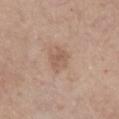No biopsy was performed on this lesion — it was imaged during a full skin examination and was not determined to be concerning.
On the chest.
The subject is a male aged approximately 60.
Approximately 3 mm at its widest.
Cropped from a total-body skin-imaging series; the visible field is about 15 mm.
The tile uses white-light illumination.